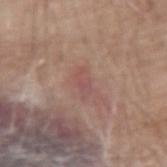Assessment: Recorded during total-body skin imaging; not selected for excision or biopsy. Background: Located on the left forearm. The total-body-photography lesion software estimated a lesion color around L≈51 a*≈22 b*≈22 in CIELAB, roughly 6 lightness units darker than nearby skin, and a normalized border contrast of about 4.5. It also reported border irregularity of about 4.5 on a 0–10 scale and a peripheral color-asymmetry measure near 0. And it measured lesion-presence confidence of about 100/100. This is a white-light tile. A male subject, in their mid- to late 60s. A 15 mm close-up tile from a total-body photography series done for melanoma screening. The recorded lesion diameter is about 3 mm.